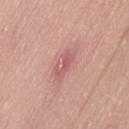<case>
  <biopsy_status>not biopsied; imaged during a skin examination</biopsy_status>
  <image>
    <source>total-body photography crop</source>
    <field_of_view_mm>15</field_of_view_mm>
  </image>
  <lesion_size>
    <long_diameter_mm_approx>3.5</long_diameter_mm_approx>
  </lesion_size>
  <patient>
    <sex>male</sex>
    <age_approx>50</age_approx>
  </patient>
  <site>lower back</site>
  <lighting>white-light</lighting>
</case>